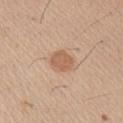The lesion was tiled from a total-body skin photograph and was not biopsied. A roughly 15 mm field-of-view crop from a total-body skin photograph. A male patient, approximately 60 years of age. From the right upper arm.